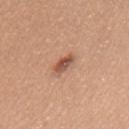Part of a total-body skin-imaging series; this lesion was reviewed on a skin check and was not flagged for biopsy. The patient is a female aged 53 to 57. Located on the upper back. A 15 mm close-up tile from a total-body photography series done for melanoma screening. Captured under white-light illumination.